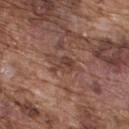No biopsy was performed on this lesion — it was imaged during a full skin examination and was not determined to be concerning. A male patient, in their mid-70s. On the upper back. This image is a 15 mm lesion crop taken from a total-body photograph.